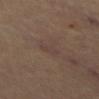Imaged during a routine full-body skin examination; the lesion was not biopsied and no histopathology is available. About 3 mm across. An algorithmic analysis of the crop reported an eccentricity of roughly 0.9 and a shape-asymmetry score of about 0.3 (0 = symmetric). The software also gave a lesion color around L≈40 a*≈15 b*≈20 in CIELAB and a lesion–skin lightness drop of about 4. The analysis additionally found a color-variation rating of about 1/10 and a peripheral color-asymmetry measure near 0. This is a cross-polarized tile. A lesion tile, about 15 mm wide, cut from a 3D total-body photograph. On the mid back.